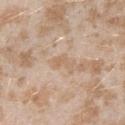No biopsy was performed on this lesion — it was imaged during a full skin examination and was not determined to be concerning.
The lesion-visualizer software estimated an outline eccentricity of about 0.85 (0 = round, 1 = elongated) and a shape-asymmetry score of about 0.45 (0 = symmetric). It also reported a lesion color around L≈63 a*≈16 b*≈32 in CIELAB, a lesion–skin lightness drop of about 6, and a normalized border contrast of about 5. It also reported a nevus-likeness score of about 0/100 and a lesion-detection confidence of about 100/100.
A female subject, roughly 25 years of age.
A lesion tile, about 15 mm wide, cut from a 3D total-body photograph.
Located on the left upper arm.
Approximately 2.5 mm at its widest.
Imaged with white-light lighting.